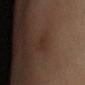Clinical impression: The lesion was tiled from a total-body skin photograph and was not biopsied. Context: A female subject, roughly 70 years of age. From the left lower leg. About 1 mm across. A region of skin cropped from a whole-body photographic capture, roughly 15 mm wide.